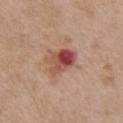Q: Was this lesion biopsied?
A: catalogued during a skin exam; not biopsied